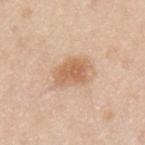This lesion was catalogued during total-body skin photography and was not selected for biopsy.
Imaged with white-light lighting.
Located on the left upper arm.
Cropped from a whole-body photographic skin survey; the tile spans about 15 mm.
The patient is a male roughly 35 years of age.